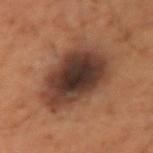No biopsy was performed on this lesion — it was imaged during a full skin examination and was not determined to be concerning.
Cropped from a total-body skin-imaging series; the visible field is about 15 mm.
The tile uses cross-polarized illumination.
From the left upper arm.
Automated image analysis of the tile measured a nevus-likeness score of about 30/100 and lesion-presence confidence of about 100/100.
The patient is a male approximately 50 years of age.